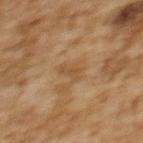The lesion was photographed on a routine skin check and not biopsied; there is no pathology result. A female subject in their 60s. The lesion-visualizer software estimated an average lesion color of about L≈43 a*≈15 b*≈32 (CIELAB), about 6 CIELAB-L* units darker than the surrounding skin, and a lesion-to-skin contrast of about 5.5 (normalized; higher = more distinct). It also reported an automated nevus-likeness rating near 0 out of 100 and lesion-presence confidence of about 100/100. The lesion is on the upper back. A lesion tile, about 15 mm wide, cut from a 3D total-body photograph. Longest diameter approximately 3 mm. The tile uses cross-polarized illumination.